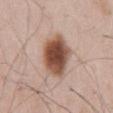Captured during whole-body skin photography for melanoma surveillance; the lesion was not biopsied.
Automated tile analysis of the lesion measured a lesion area of about 16 mm² and two-axis asymmetry of about 0.15. It also reported a peripheral color-asymmetry measure near 1.5.
A male patient approximately 55 years of age.
From the abdomen.
A roughly 15 mm field-of-view crop from a total-body skin photograph.
Imaged with white-light lighting.